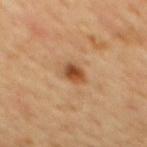Q: Is there a histopathology result?
A: total-body-photography surveillance lesion; no biopsy
Q: What are the patient's age and sex?
A: male, aged 58–62
Q: How was this image acquired?
A: ~15 mm tile from a whole-body skin photo
Q: How large is the lesion?
A: ~2.5 mm (longest diameter)
Q: Lesion location?
A: the mid back
Q: How was the tile lit?
A: cross-polarized illumination